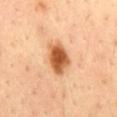Assessment: The lesion was photographed on a routine skin check and not biopsied; there is no pathology result. Background: On the mid back. A 15 mm close-up extracted from a 3D total-body photography capture. The subject is a male approximately 35 years of age.